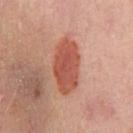Notes:
– workup · no biopsy performed (imaged during a skin exam)
– image source · ~15 mm crop, total-body skin-cancer survey
– illumination · cross-polarized
– patient · female, in their 50s
– anatomic site · the left thigh
– image-analysis metrics · a mean CIELAB color near L≈50 a*≈28 b*≈30